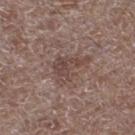Q: Is there a histopathology result?
A: total-body-photography surveillance lesion; no biopsy
Q: Who is the patient?
A: male, aged 68–72
Q: How was the tile lit?
A: white-light
Q: Lesion size?
A: ≈4.5 mm
Q: What is the imaging modality?
A: 15 mm crop, total-body photography
Q: Where on the body is the lesion?
A: the left lower leg